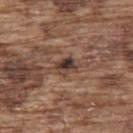{
  "biopsy_status": "not biopsied; imaged during a skin examination",
  "lesion_size": {
    "long_diameter_mm_approx": 3.0
  },
  "image": {
    "source": "total-body photography crop",
    "field_of_view_mm": 15
  },
  "site": "upper back",
  "lighting": "white-light",
  "patient": {
    "sex": "male",
    "age_approx": 75
  },
  "automated_metrics": {
    "eccentricity": 0.65,
    "cielab_L": 39,
    "cielab_a": 17,
    "cielab_b": 23,
    "vs_skin_contrast_norm": 10.0
  }
}